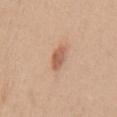{
  "biopsy_status": "not biopsied; imaged during a skin examination",
  "image": {
    "source": "total-body photography crop",
    "field_of_view_mm": 15
  },
  "patient": {
    "sex": "male",
    "age_approx": 30
  },
  "automated_metrics": {
    "area_mm2_approx": 4.5,
    "eccentricity": 0.8,
    "shape_asymmetry": 0.2,
    "cielab_L": 59,
    "cielab_a": 23,
    "cielab_b": 32,
    "vs_skin_darker_L": 11.0,
    "vs_skin_contrast_norm": 7.5,
    "color_variation_0_10": 2.5,
    "nevus_likeness_0_100": 95,
    "lesion_detection_confidence_0_100": 100
  },
  "site": "back",
  "lighting": "white-light"
}